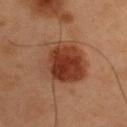The subject is a male roughly 55 years of age. Captured under cross-polarized illumination. Automated tile analysis of the lesion measured a lesion area of about 24 mm², an eccentricity of roughly 0.35, and a shape-asymmetry score of about 0.15 (0 = symmetric). The analysis additionally found a lesion color around L≈31 a*≈21 b*≈26 in CIELAB and about 10 CIELAB-L* units darker than the surrounding skin. The lesion is on the right upper arm. This image is a 15 mm lesion crop taken from a total-body photograph.